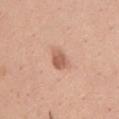Part of a total-body skin-imaging series; this lesion was reviewed on a skin check and was not flagged for biopsy.
Imaged with white-light lighting.
An algorithmic analysis of the crop reported a color-variation rating of about 2.5/10. It also reported a classifier nevus-likeness of about 75/100 and lesion-presence confidence of about 100/100.
Located on the chest.
A close-up tile cropped from a whole-body skin photograph, about 15 mm across.
About 2.5 mm across.
The subject is a female aged 38–42.